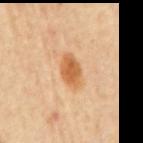The lesion was photographed on a routine skin check and not biopsied; there is no pathology result. The lesion-visualizer software estimated an area of roughly 8 mm², an eccentricity of roughly 0.85, and a symmetry-axis asymmetry near 0.2. The analysis additionally found a lesion color around L≈60 a*≈23 b*≈41 in CIELAB, roughly 12 lightness units darker than nearby skin, and a lesion-to-skin contrast of about 8.5 (normalized; higher = more distinct). The analysis additionally found a border-irregularity index near 2/10 and peripheral color asymmetry of about 1. And it measured lesion-presence confidence of about 100/100. The patient is a female approximately 65 years of age. The lesion is located on the mid back. The lesion's longest dimension is about 4.5 mm. The tile uses cross-polarized illumination. A close-up tile cropped from a whole-body skin photograph, about 15 mm across.